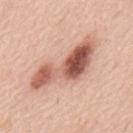Imaged during a routine full-body skin examination; the lesion was not biopsied and no histopathology is available.
The lesion is located on the upper back.
A male subject aged 63–67.
Longest diameter approximately 9 mm.
This is a white-light tile.
Automated tile analysis of the lesion measured a lesion area of about 20 mm² and an eccentricity of roughly 0.95. The analysis additionally found a mean CIELAB color near L≈58 a*≈25 b*≈29, about 17 CIELAB-L* units darker than the surrounding skin, and a lesion-to-skin contrast of about 10.5 (normalized; higher = more distinct). The analysis additionally found a border-irregularity index near 6.5/10, a within-lesion color-variation index near 9.5/10, and peripheral color asymmetry of about 3.5. The analysis additionally found a classifier nevus-likeness of about 90/100 and a lesion-detection confidence of about 100/100.
A roughly 15 mm field-of-view crop from a total-body skin photograph.